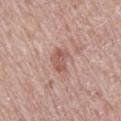notes — no biopsy performed (imaged during a skin exam) | anatomic site — the right thigh | imaging modality — 15 mm crop, total-body photography | subject — male, aged approximately 70 | automated lesion analysis — a footprint of about 5 mm² and an eccentricity of roughly 0.7; a lesion–skin lightness drop of about 10 and a lesion-to-skin contrast of about 6.5 (normalized; higher = more distinct); an automated nevus-likeness rating near 5 out of 100 | lesion diameter — about 3 mm.